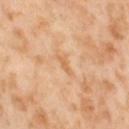This lesion was catalogued during total-body skin photography and was not selected for biopsy. Captured under cross-polarized illumination. A 15 mm close-up tile from a total-body photography series done for melanoma screening. Located on the leg. A female subject in their mid-50s.